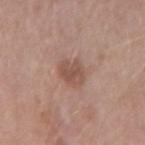Q: Is there a histopathology result?
A: total-body-photography surveillance lesion; no biopsy
Q: What kind of image is this?
A: ~15 mm crop, total-body skin-cancer survey
Q: What is the anatomic site?
A: the left forearm
Q: Who is the patient?
A: female, roughly 45 years of age
Q: Automated lesion metrics?
A: an average lesion color of about L≈51 a*≈20 b*≈26 (CIELAB) and a normalized border contrast of about 6.5
Q: How was the tile lit?
A: white-light
Q: What is the lesion's diameter?
A: ≈3 mm